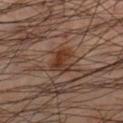biopsy status = total-body-photography surveillance lesion; no biopsy | acquisition = total-body-photography crop, ~15 mm field of view | automated lesion analysis = a mean CIELAB color near L≈35 a*≈20 b*≈28, a lesion–skin lightness drop of about 9, and a normalized border contrast of about 9 | location = the leg | illumination = cross-polarized | subject = male, roughly 50 years of age.